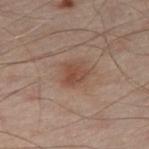The lesion was tiled from a total-body skin photograph and was not biopsied. A male patient approximately 50 years of age. The lesion is on the left leg. A 15 mm close-up extracted from a 3D total-body photography capture.